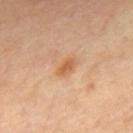Part of a total-body skin-imaging series; this lesion was reviewed on a skin check and was not flagged for biopsy. About 2.5 mm across. A male subject, aged approximately 65. A 15 mm crop from a total-body photograph taken for skin-cancer surveillance. From the mid back.